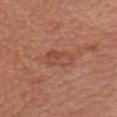follow-up: imaged on a skin check; not biopsied | image source: ~15 mm crop, total-body skin-cancer survey | patient: female, aged 63–67 | anatomic site: the chest.